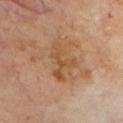biopsy status — no biopsy performed (imaged during a skin exam) | diameter — ≈5.5 mm | image — ~15 mm crop, total-body skin-cancer survey | location — the front of the torso | tile lighting — cross-polarized illumination | patient — male, approximately 70 years of age.